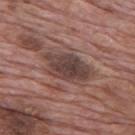Clinical impression:
Recorded during total-body skin imaging; not selected for excision or biopsy.
Acquisition and patient details:
The patient is a male in their 70s. Captured under white-light illumination. Automated image analysis of the tile measured an average lesion color of about L≈41 a*≈17 b*≈20 (CIELAB), about 11 CIELAB-L* units darker than the surrounding skin, and a normalized lesion–skin contrast near 9. The analysis additionally found border irregularity of about 3 on a 0–10 scale, internal color variation of about 4.5 on a 0–10 scale, and radial color variation of about 1. A close-up tile cropped from a whole-body skin photograph, about 15 mm across. The recorded lesion diameter is about 6 mm. The lesion is located on the mid back.